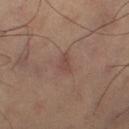Case summary:
* biopsy status — catalogued during a skin exam; not biopsied
* subject — male, aged approximately 60
* anatomic site — the right thigh
* imaging modality — 15 mm crop, total-body photography